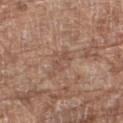  biopsy_status: not biopsied; imaged during a skin examination
  image:
    source: total-body photography crop
    field_of_view_mm: 15
  lesion_size:
    long_diameter_mm_approx: 3.0
  lighting: white-light
  site: right thigh
  patient:
    sex: female
    age_approx: 75